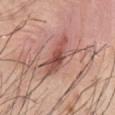follow-up = catalogued during a skin exam; not biopsied
image source = 15 mm crop, total-body photography
body site = the front of the torso
illumination = white-light
size = ~5.5 mm (longest diameter)
subject = male, in their mid-40s
TBP lesion metrics = a mean CIELAB color near L≈52 a*≈25 b*≈26, a lesion–skin lightness drop of about 12, and a normalized lesion–skin contrast near 8; a nevus-likeness score of about 10/100 and a lesion-detection confidence of about 100/100